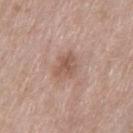Captured during whole-body skin photography for melanoma surveillance; the lesion was not biopsied.